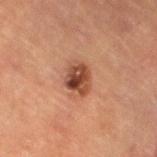Located on the left thigh. Captured under cross-polarized illumination. The lesion-visualizer software estimated a mean CIELAB color near L≈41 a*≈22 b*≈28 and a lesion-to-skin contrast of about 9.5 (normalized; higher = more distinct). The analysis additionally found a border-irregularity rating of about 2/10, a color-variation rating of about 7/10, and radial color variation of about 2.5. Measured at roughly 3.5 mm in maximum diameter. A male subject approximately 85 years of age or older. A roughly 15 mm field-of-view crop from a total-body skin photograph.